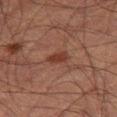Findings:
– subject: male, approximately 50 years of age
– image source: ~15 mm tile from a whole-body skin photo
– location: the right thigh